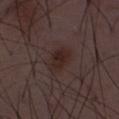Clinical summary:
A roughly 15 mm field-of-view crop from a total-body skin photograph. Captured under white-light illumination. About 4 mm across. A male patient aged 48 to 52.